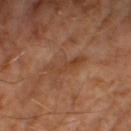follow-up = imaged on a skin check; not biopsied
acquisition = ~15 mm tile from a whole-body skin photo
subject = male, about 65 years old